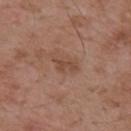The lesion is located on the back. Cropped from a whole-body photographic skin survey; the tile spans about 15 mm. The patient is a male about 55 years old. Automated image analysis of the tile measured a footprint of about 3.5 mm², an outline eccentricity of about 0.9 (0 = round, 1 = elongated), and two-axis asymmetry of about 0.45. The analysis additionally found an average lesion color of about L≈46 a*≈20 b*≈28 (CIELAB), a lesion–skin lightness drop of about 8, and a lesion-to-skin contrast of about 6 (normalized; higher = more distinct). The software also gave a color-variation rating of about 0.5/10 and peripheral color asymmetry of about 0. It also reported a detector confidence of about 100 out of 100 that the crop contains a lesion.